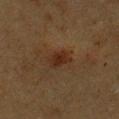The lesion was photographed on a routine skin check and not biopsied; there is no pathology result. The lesion-visualizer software estimated a footprint of about 4 mm², a shape eccentricity near 0.65, and a shape-asymmetry score of about 0.2 (0 = symmetric). It also reported an average lesion color of about L≈21 a*≈16 b*≈23 (CIELAB) and a lesion-to-skin contrast of about 8 (normalized; higher = more distinct). The analysis additionally found a border-irregularity rating of about 2/10, a color-variation rating of about 1.5/10, and peripheral color asymmetry of about 0.5. Captured under cross-polarized illumination. Located on the chest. Cropped from a total-body skin-imaging series; the visible field is about 15 mm. Measured at roughly 2.5 mm in maximum diameter. A male patient, aged 73–77.